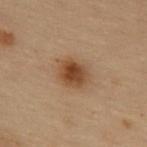Q: Is there a histopathology result?
A: total-body-photography surveillance lesion; no biopsy
Q: What kind of image is this?
A: 15 mm crop, total-body photography
Q: What lighting was used for the tile?
A: cross-polarized illumination
Q: Lesion size?
A: ~3.5 mm (longest diameter)
Q: Automated lesion metrics?
A: an area of roughly 8 mm² and a shape-asymmetry score of about 0.15 (0 = symmetric); an automated nevus-likeness rating near 100 out of 100
Q: Where on the body is the lesion?
A: the abdomen
Q: Patient demographics?
A: male, aged 53 to 57